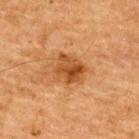The lesion was tiled from a total-body skin photograph and was not biopsied.
A male subject aged 63 to 67.
The lesion is on the upper back.
Cropped from a whole-body photographic skin survey; the tile spans about 15 mm.
Imaged with cross-polarized lighting.
The lesion's longest dimension is about 3.5 mm.
The total-body-photography lesion software estimated a footprint of about 9 mm² and an eccentricity of roughly 0.55. It also reported a nevus-likeness score of about 75/100.